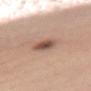lighting: white-light
patient: female, in their mid-30s
automated metrics: roughly 13 lightness units darker than nearby skin and a normalized lesion–skin contrast near 9; an automated nevus-likeness rating near 90 out of 100 and lesion-presence confidence of about 100/100
acquisition: ~15 mm tile from a whole-body skin photo
location: the chest